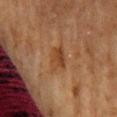Q: Was this lesion biopsied?
A: catalogued during a skin exam; not biopsied
Q: Lesion size?
A: about 3 mm
Q: What lighting was used for the tile?
A: cross-polarized illumination
Q: Patient demographics?
A: male, aged around 80
Q: What kind of image is this?
A: ~15 mm crop, total-body skin-cancer survey
Q: Lesion location?
A: the abdomen
Q: What did automated image analysis measure?
A: a mean CIELAB color near L≈33 a*≈18 b*≈29 and roughly 6 lightness units darker than nearby skin; a nevus-likeness score of about 0/100 and a detector confidence of about 100 out of 100 that the crop contains a lesion